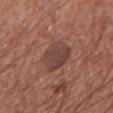The lesion was photographed on a routine skin check and not biopsied; there is no pathology result.
This is a white-light tile.
Located on the front of the torso.
A female patient aged 73–77.
The total-body-photography lesion software estimated a classifier nevus-likeness of about 5/100.
This image is a 15 mm lesion crop taken from a total-body photograph.
The lesion's longest dimension is about 5 mm.